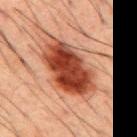Recorded during total-body skin imaging; not selected for excision or biopsy. About 9 mm across. A male patient approximately 50 years of age. Imaged with cross-polarized lighting. From the back. A lesion tile, about 15 mm wide, cut from a 3D total-body photograph.